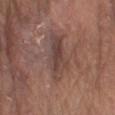A male subject, about 80 years old.
This is a white-light tile.
Located on the left upper arm.
Approximately 5.5 mm at its widest.
A roughly 15 mm field-of-view crop from a total-body skin photograph.